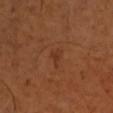Context: Longest diameter approximately 2.5 mm. The lesion-visualizer software estimated an area of roughly 2.5 mm². And it measured a lesion–skin lightness drop of about 5. The subject is a male about 50 years old. A roughly 15 mm field-of-view crop from a total-body skin photograph. From the left upper arm.